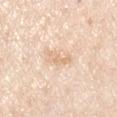Part of a total-body skin-imaging series; this lesion was reviewed on a skin check and was not flagged for biopsy. A male patient, in their mid- to late 30s. Cropped from a total-body skin-imaging series; the visible field is about 15 mm. An algorithmic analysis of the crop reported an average lesion color of about L≈75 a*≈16 b*≈34 (CIELAB) and roughly 8 lightness units darker than nearby skin. The analysis additionally found a nevus-likeness score of about 0/100. The tile uses white-light illumination. About 3.5 mm across. Located on the left upper arm.